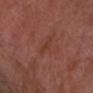Recorded during total-body skin imaging; not selected for excision or biopsy.
A 15 mm crop from a total-body photograph taken for skin-cancer surveillance.
The lesion is located on the left forearm.
Approximately 2.5 mm at its widest.
The lesion-visualizer software estimated an area of roughly 3 mm², an eccentricity of roughly 0.85, and a shape-asymmetry score of about 0.25 (0 = symmetric). It also reported border irregularity of about 2.5 on a 0–10 scale and internal color variation of about 1 on a 0–10 scale.
A male patient in their 30s.
Captured under cross-polarized illumination.